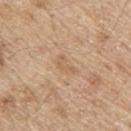The lesion was tiled from a total-body skin photograph and was not biopsied.
This is a white-light tile.
The total-body-photography lesion software estimated a footprint of about 4 mm², an outline eccentricity of about 0.85 (0 = round, 1 = elongated), and a symmetry-axis asymmetry near 0.4. The analysis additionally found an average lesion color of about L≈61 a*≈17 b*≈34 (CIELAB), about 6 CIELAB-L* units darker than the surrounding skin, and a lesion-to-skin contrast of about 4.5 (normalized; higher = more distinct). The software also gave a border-irregularity rating of about 4.5/10, a within-lesion color-variation index near 1.5/10, and radial color variation of about 0.5. It also reported a classifier nevus-likeness of about 0/100 and a lesion-detection confidence of about 100/100.
A region of skin cropped from a whole-body photographic capture, roughly 15 mm wide.
The recorded lesion diameter is about 3 mm.
From the upper back.
A male subject aged approximately 70.